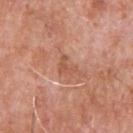follow-up: catalogued during a skin exam; not biopsied
size: ~2.5 mm (longest diameter)
tile lighting: white-light
patient: male, about 55 years old
imaging modality: ~15 mm tile from a whole-body skin photo
location: the back